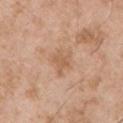No biopsy was performed on this lesion — it was imaged during a full skin examination and was not determined to be concerning. A male patient, about 55 years old. The lesion-visualizer software estimated an area of roughly 5 mm² and a shape-asymmetry score of about 0.5 (0 = symmetric). The analysis additionally found internal color variation of about 2 on a 0–10 scale and peripheral color asymmetry of about 0.5. It also reported a detector confidence of about 100 out of 100 that the crop contains a lesion. The lesion is on the upper back. Cropped from a total-body skin-imaging series; the visible field is about 15 mm. Captured under white-light illumination.